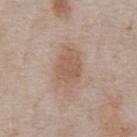<lesion>
<biopsy_status>not biopsied; imaged during a skin examination</biopsy_status>
<patient>
  <sex>male</sex>
  <age_approx>75</age_approx>
</patient>
<lesion_size>
  <long_diameter_mm_approx>5.5</long_diameter_mm_approx>
</lesion_size>
<image>
  <source>total-body photography crop</source>
  <field_of_view_mm>15</field_of_view_mm>
</image>
<automated_metrics>
  <border_irregularity_0_10>3.0</border_irregularity_0_10>
  <color_variation_0_10>2.5</color_variation_0_10>
  <peripheral_color_asymmetry>0.5</peripheral_color_asymmetry>
</automated_metrics>
<lighting>white-light</lighting>
<site>front of the torso</site>
</lesion>